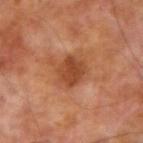notes: total-body-photography surveillance lesion; no biopsy
lighting: cross-polarized illumination
acquisition: 15 mm crop, total-body photography
body site: the right upper arm
patient: male, aged around 70
lesion diameter: about 3.5 mm
automated lesion analysis: a lesion area of about 8.5 mm², a shape eccentricity near 0.55, and two-axis asymmetry of about 0.15